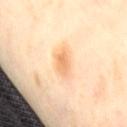Impression:
This lesion was catalogued during total-body skin photography and was not selected for biopsy.
Context:
This is a cross-polarized tile. The patient is a female in their 50s. A region of skin cropped from a whole-body photographic capture, roughly 15 mm wide. Automated image analysis of the tile measured a border-irregularity index near 2/10 and a color-variation rating of about 3/10. On the back.